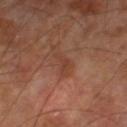From the right lower leg. Measured at roughly 3 mm in maximum diameter. The total-body-photography lesion software estimated an area of roughly 3 mm² and an eccentricity of roughly 0.9. The analysis additionally found an automated nevus-likeness rating near 0 out of 100 and a lesion-detection confidence of about 100/100. The tile uses cross-polarized illumination. A roughly 15 mm field-of-view crop from a total-body skin photograph. A male subject in their 70s.